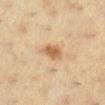{
  "biopsy_status": "not biopsied; imaged during a skin examination",
  "site": "left lower leg",
  "patient": {
    "sex": "female",
    "age_approx": 40
  },
  "image": {
    "source": "total-body photography crop",
    "field_of_view_mm": 15
  }
}